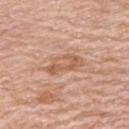{"biopsy_status": "not biopsied; imaged during a skin examination"}